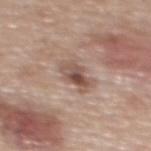  biopsy_status: not biopsied; imaged during a skin examination
  automated_metrics:
    area_mm2_approx: 7.0
    eccentricity: 0.7
    shape_asymmetry: 0.3
    cielab_L: 52
    cielab_a: 18
    cielab_b: 25
    vs_skin_darker_L: 12.0
    vs_skin_contrast_norm: 8.0
    border_irregularity_0_10: 3.5
    color_variation_0_10: 7.5
    peripheral_color_asymmetry: 2.5
    nevus_likeness_0_100: 55
    lesion_detection_confidence_0_100: 100
  patient:
    sex: female
    age_approx: 60
  lesion_size:
    long_diameter_mm_approx: 3.5
  image:
    source: total-body photography crop
    field_of_view_mm: 15
  site: upper back
  lighting: white-light